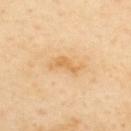Captured during whole-body skin photography for melanoma surveillance; the lesion was not biopsied.